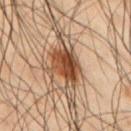Part of a total-body skin-imaging series; this lesion was reviewed on a skin check and was not flagged for biopsy. Captured under cross-polarized illumination. The lesion is located on the right upper arm. The patient is a male aged approximately 50. A lesion tile, about 15 mm wide, cut from a 3D total-body photograph.